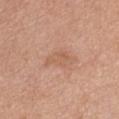– image source — ~15 mm tile from a whole-body skin photo
– subject — female, approximately 45 years of age
– TBP lesion metrics — a shape eccentricity near 0.85; roughly 6 lightness units darker than nearby skin and a normalized border contrast of about 5
– lesion diameter — ≈3.5 mm
– body site — the chest